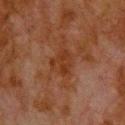Part of a total-body skin-imaging series; this lesion was reviewed on a skin check and was not flagged for biopsy.
The lesion-visualizer software estimated a lesion area of about 5.5 mm², an outline eccentricity of about 0.5 (0 = round, 1 = elongated), and a shape-asymmetry score of about 0.5 (0 = symmetric). The software also gave a lesion color around L≈27 a*≈20 b*≈27 in CIELAB, a lesion–skin lightness drop of about 5, and a normalized lesion–skin contrast near 6. The software also gave a border-irregularity rating of about 6/10, a within-lesion color-variation index near 2/10, and peripheral color asymmetry of about 0.5.
Cropped from a total-body skin-imaging series; the visible field is about 15 mm.
Located on the upper back.
Imaged with cross-polarized lighting.
A male subject, aged around 80.